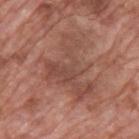The lesion was photographed on a routine skin check and not biopsied; there is no pathology result.
On the upper back.
The patient is a male aged approximately 70.
A 15 mm crop from a total-body photograph taken for skin-cancer surveillance.
Imaged with white-light lighting.